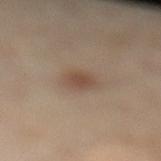biopsy status: total-body-photography surveillance lesion; no biopsy
lesion diameter: ~2.5 mm (longest diameter)
image-analysis metrics: a mean CIELAB color near L≈48 a*≈16 b*≈27, roughly 9 lightness units darker than nearby skin, and a normalized border contrast of about 7; a border-irregularity rating of about 1.5/10 and a peripheral color-asymmetry measure near 0.5; a classifier nevus-likeness of about 85/100 and a detector confidence of about 100 out of 100 that the crop contains a lesion
image: 15 mm crop, total-body photography
illumination: cross-polarized illumination
body site: the left lower leg
patient: female, roughly 45 years of age